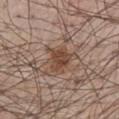biopsy status: total-body-photography surveillance lesion; no biopsy.